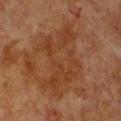{
  "biopsy_status": "not biopsied; imaged during a skin examination",
  "lighting": "cross-polarized",
  "image": {
    "source": "total-body photography crop",
    "field_of_view_mm": 15
  },
  "patient": {
    "sex": "female",
    "age_approx": 55
  },
  "lesion_size": {
    "long_diameter_mm_approx": 9.0
  },
  "site": "chest"
}